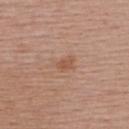biopsy_status: not biopsied; imaged during a skin examination
patient:
  sex: female
  age_approx: 55
image:
  source: total-body photography crop
  field_of_view_mm: 15
site: back